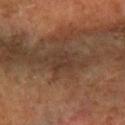Recorded during total-body skin imaging; not selected for excision or biopsy.
A roughly 15 mm field-of-view crop from a total-body skin photograph.
The lesion is on the right forearm.
A female subject, about 60 years old.
The tile uses cross-polarized illumination.